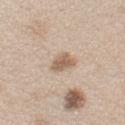Clinical impression:
No biopsy was performed on this lesion — it was imaged during a full skin examination and was not determined to be concerning.
Image and clinical context:
A roughly 15 mm field-of-view crop from a total-body skin photograph. From the left upper arm. The subject is a female in their mid- to late 40s.